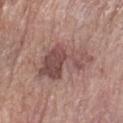| key | value |
|---|---|
| notes | catalogued during a skin exam; not biopsied |
| tile lighting | white-light |
| site | the left forearm |
| image | ~15 mm crop, total-body skin-cancer survey |
| lesion diameter | ~6.5 mm (longest diameter) |
| patient | male, aged approximately 75 |